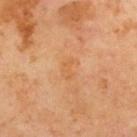Q: Is there a histopathology result?
A: no biopsy performed (imaged during a skin exam)